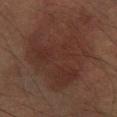<tbp_lesion>
<biopsy_status>not biopsied; imaged during a skin examination</biopsy_status>
<lesion_size>
  <long_diameter_mm_approx>8.0</long_diameter_mm_approx>
</lesion_size>
<site>arm</site>
<lighting>cross-polarized</lighting>
<image>
  <source>total-body photography crop</source>
  <field_of_view_mm>15</field_of_view_mm>
</image>
<patient>
  <sex>male</sex>
  <age_approx>85+</age_approx>
</patient>
</tbp_lesion>